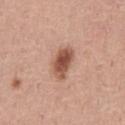  biopsy_status: not biopsied; imaged during a skin examination
  patient:
    sex: male
    age_approx: 55
  lighting: white-light
  automated_metrics:
    area_mm2_approx: 7.5
    eccentricity: 0.8
    shape_asymmetry: 0.2
  image:
    source: total-body photography crop
    field_of_view_mm: 15
  lesion_size:
    long_diameter_mm_approx: 4.0
  site: chest